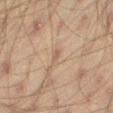This lesion was catalogued during total-body skin photography and was not selected for biopsy. Cropped from a total-body skin-imaging series; the visible field is about 15 mm. The lesion is located on the right thigh. Longest diameter approximately 2.5 mm. A male patient approximately 45 years of age. Captured under cross-polarized illumination.